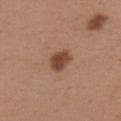Q: Was this lesion biopsied?
A: catalogued during a skin exam; not biopsied
Q: What is the lesion's diameter?
A: about 3 mm
Q: What kind of image is this?
A: ~15 mm crop, total-body skin-cancer survey
Q: What did automated image analysis measure?
A: a shape-asymmetry score of about 0.2 (0 = symmetric); an average lesion color of about L≈45 a*≈21 b*≈30 (CIELAB), about 13 CIELAB-L* units darker than the surrounding skin, and a normalized border contrast of about 9.5; lesion-presence confidence of about 100/100
Q: Where on the body is the lesion?
A: the upper back
Q: Patient demographics?
A: male, aged approximately 40